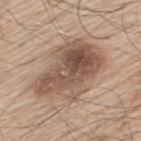biopsy_status: not biopsied; imaged during a skin examination
lesion_size:
  long_diameter_mm_approx: 8.0
lighting: white-light
patient:
  sex: male
  age_approx: 70
site: upper back
image:
  source: total-body photography crop
  field_of_view_mm: 15
automated_metrics:
  cielab_L: 53
  cielab_a: 17
  cielab_b: 27
  vs_skin_darker_L: 13.0
  color_variation_0_10: 8.0
  peripheral_color_asymmetry: 3.0
  lesion_detection_confidence_0_100: 100